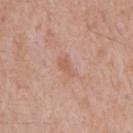The lesion was tiled from a total-body skin photograph and was not biopsied.
A close-up tile cropped from a whole-body skin photograph, about 15 mm across.
From the mid back.
A male patient aged 58 to 62.